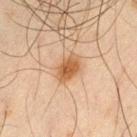{"image": {"source": "total-body photography crop", "field_of_view_mm": 15}, "patient": {"sex": "male", "age_approx": 45}, "site": "left thigh"}